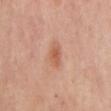workup=catalogued during a skin exam; not biopsied | illumination=cross-polarized illumination | body site=the mid back | size=≈3 mm | subject=male, in their mid-60s | image source=15 mm crop, total-body photography.